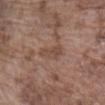Case summary:
• biopsy status: total-body-photography surveillance lesion; no biopsy
• subject: male, aged 73 to 77
• lesion diameter: ~3.5 mm (longest diameter)
• illumination: white-light
• anatomic site: the front of the torso
• image source: ~15 mm tile from a whole-body skin photo